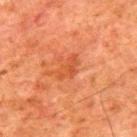Impression:
Recorded during total-body skin imaging; not selected for excision or biopsy.
Context:
Imaged with cross-polarized lighting. The recorded lesion diameter is about 3.5 mm. The subject is a male aged approximately 80. A region of skin cropped from a whole-body photographic capture, roughly 15 mm wide. Located on the upper back.